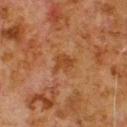Clinical impression:
Recorded during total-body skin imaging; not selected for excision or biopsy.
Image and clinical context:
The lesion is located on the upper back. A male patient approximately 80 years of age. Captured under cross-polarized illumination. Cropped from a whole-body photographic skin survey; the tile spans about 15 mm. Measured at roughly 3 mm in maximum diameter.